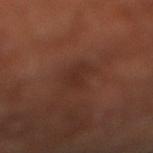Assessment:
Part of a total-body skin-imaging series; this lesion was reviewed on a skin check and was not flagged for biopsy.
Clinical summary:
From the right lower leg. Cropped from a whole-body photographic skin survey; the tile spans about 15 mm. A male subject, roughly 70 years of age.